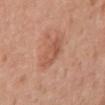Assessment: This lesion was catalogued during total-body skin photography and was not selected for biopsy. Image and clinical context: A region of skin cropped from a whole-body photographic capture, roughly 15 mm wide. Imaged with white-light lighting. On the right upper arm. A female patient, in their mid-50s. Measured at roughly 3.5 mm in maximum diameter.